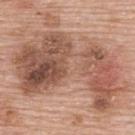Case summary:
• workup: imaged on a skin check; not biopsied
• TBP lesion metrics: a mean CIELAB color near L≈54 a*≈21 b*≈28 and a normalized border contrast of about 8
• imaging modality: ~15 mm tile from a whole-body skin photo
• site: the upper back
• lighting: white-light illumination
• lesion size: about 13.5 mm
• subject: female, about 60 years old